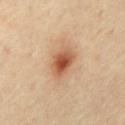notes: imaged on a skin check; not biopsied
tile lighting: cross-polarized
automated lesion analysis: a lesion color around L≈56 a*≈23 b*≈35 in CIELAB, a lesion–skin lightness drop of about 14, and a normalized border contrast of about 9; border irregularity of about 2 on a 0–10 scale, a color-variation rating of about 7/10, and radial color variation of about 1.5; a classifier nevus-likeness of about 95/100 and a detector confidence of about 100 out of 100 that the crop contains a lesion
image source: 15 mm crop, total-body photography
lesion diameter: about 4.5 mm
patient: male, aged around 65
location: the chest